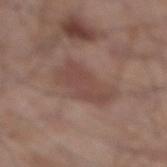Clinical impression: No biopsy was performed on this lesion — it was imaged during a full skin examination and was not determined to be concerning. Acquisition and patient details: About 4.5 mm across. The lesion is located on the right lower leg. The tile uses white-light illumination. A region of skin cropped from a whole-body photographic capture, roughly 15 mm wide. A male patient, aged around 55.